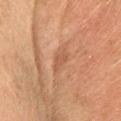follow-up — catalogued during a skin exam; not biopsied
automated metrics — a within-lesion color-variation index near 0.5/10 and radial color variation of about 0; a nevus-likeness score of about 0/100 and a detector confidence of about 80 out of 100 that the crop contains a lesion
lighting — cross-polarized illumination
anatomic site — the head or neck
patient — female, aged 58 to 62
image — ~15 mm tile from a whole-body skin photo
lesion diameter — about 3 mm